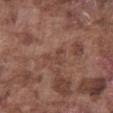Case summary:
• workup: total-body-photography surveillance lesion; no biopsy
• subject: male, in their mid-70s
• site: the front of the torso
• image source: total-body-photography crop, ~15 mm field of view